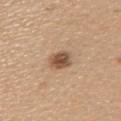No biopsy was performed on this lesion — it was imaged during a full skin examination and was not determined to be concerning.
The tile uses white-light illumination.
A 15 mm close-up tile from a total-body photography series done for melanoma screening.
The recorded lesion diameter is about 3 mm.
A female subject, roughly 60 years of age.
The lesion is on the upper back.